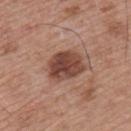The lesion was photographed on a routine skin check and not biopsied; there is no pathology result. Cropped from a total-body skin-imaging series; the visible field is about 15 mm. From the upper back. Captured under white-light illumination. The subject is a male aged around 65. Automated tile analysis of the lesion measured a border-irregularity index near 1.5/10, a color-variation rating of about 5/10, and peripheral color asymmetry of about 1.5. It also reported a nevus-likeness score of about 80/100.